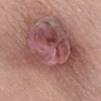| feature | finding |
|---|---|
| follow-up | no biopsy performed (imaged during a skin exam) |
| acquisition | ~15 mm crop, total-body skin-cancer survey |
| anatomic site | the abdomen |
| patient | male, approximately 50 years of age |
| lighting | white-light |
| image-analysis metrics | a lesion area of about 95 mm² and a shape-asymmetry score of about 0.3 (0 = symmetric); a lesion–skin lightness drop of about 14 and a normalized border contrast of about 9.5; a within-lesion color-variation index near 8.5/10 |